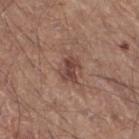Q: What is the anatomic site?
A: the right thigh
Q: Lesion size?
A: about 3 mm
Q: How was this image acquired?
A: 15 mm crop, total-body photography
Q: Automated lesion metrics?
A: a lesion area of about 4.5 mm² and two-axis asymmetry of about 0.35; border irregularity of about 3.5 on a 0–10 scale and a color-variation rating of about 2.5/10; lesion-presence confidence of about 100/100
Q: Patient demographics?
A: male, aged 63 to 67
Q: How was the tile lit?
A: white-light